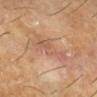A male patient aged 58 to 62. Located on the right lower leg. A lesion tile, about 15 mm wide, cut from a 3D total-body photograph. The lesion's longest dimension is about 5 mm. This is a cross-polarized tile.